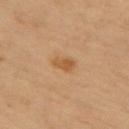Impression: This lesion was catalogued during total-body skin photography and was not selected for biopsy. Background: A female subject aged 68 to 72. A 15 mm crop from a total-body photograph taken for skin-cancer surveillance. The recorded lesion diameter is about 2.5 mm. Located on the left thigh.